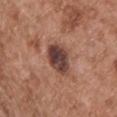Clinical impression: No biopsy was performed on this lesion — it was imaged during a full skin examination and was not determined to be concerning. Image and clinical context: A roughly 15 mm field-of-view crop from a total-body skin photograph. This is a white-light tile. The lesion-visualizer software estimated an area of roughly 9 mm², an outline eccentricity of about 0.4 (0 = round, 1 = elongated), and two-axis asymmetry of about 0.15. The analysis additionally found roughly 16 lightness units darker than nearby skin and a normalized border contrast of about 12.5. The analysis additionally found a border-irregularity index near 1.5/10, a color-variation rating of about 6/10, and a peripheral color-asymmetry measure near 2. Longest diameter approximately 3.5 mm. The lesion is on the chest. The subject is a male aged approximately 55.